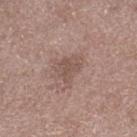| field | value |
|---|---|
| notes | total-body-photography surveillance lesion; no biopsy |
| acquisition | total-body-photography crop, ~15 mm field of view |
| location | the right lower leg |
| size | ~3 mm (longest diameter) |
| lighting | white-light |
| subject | male, aged 48–52 |